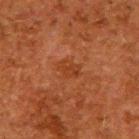Impression: Captured during whole-body skin photography for melanoma surveillance; the lesion was not biopsied. Context: The subject is a male aged around 60. Measured at roughly 3 mm in maximum diameter. On the upper back. The tile uses cross-polarized illumination. A close-up tile cropped from a whole-body skin photograph, about 15 mm across.